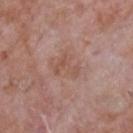The lesion was photographed on a routine skin check and not biopsied; there is no pathology result. This is a white-light tile. Automated image analysis of the tile measured an area of roughly 6 mm² and a shape-asymmetry score of about 0.6 (0 = symmetric). The analysis additionally found about 6 CIELAB-L* units darker than the surrounding skin and a normalized border contrast of about 5.5. It also reported a color-variation rating of about 1.5/10 and peripheral color asymmetry of about 0.5. The lesion's longest dimension is about 3.5 mm. From the upper back. The patient is a male aged 63–67. Cropped from a total-body skin-imaging series; the visible field is about 15 mm.